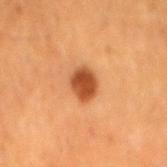Part of a total-body skin-imaging series; this lesion was reviewed on a skin check and was not flagged for biopsy. A lesion tile, about 15 mm wide, cut from a 3D total-body photograph. From the lower back. A male subject, aged around 65.